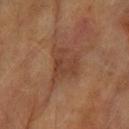Clinical impression:
Recorded during total-body skin imaging; not selected for excision or biopsy.
Background:
Automated tile analysis of the lesion measured a lesion area of about 11 mm² and a shape-asymmetry score of about 0.4 (0 = symmetric). The analysis additionally found a lesion color around L≈37 a*≈19 b*≈27 in CIELAB, about 6 CIELAB-L* units darker than the surrounding skin, and a normalized lesion–skin contrast near 6. The subject is a female aged 58 to 62. Captured under cross-polarized illumination. Cropped from a total-body skin-imaging series; the visible field is about 15 mm. The lesion is located on the left forearm. The lesion's longest dimension is about 4.5 mm.